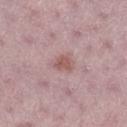{
  "biopsy_status": "not biopsied; imaged during a skin examination",
  "site": "leg",
  "patient": {
    "sex": "female",
    "age_approx": 40
  },
  "image": {
    "source": "total-body photography crop",
    "field_of_view_mm": 15
  },
  "automated_metrics": {
    "area_mm2_approx": 4.0,
    "eccentricity": 0.7,
    "shape_asymmetry": 0.2,
    "border_irregularity_0_10": 2.0,
    "color_variation_0_10": 1.5,
    "peripheral_color_asymmetry": 0.5,
    "nevus_likeness_0_100": 15
  },
  "lighting": "white-light",
  "lesion_size": {
    "long_diameter_mm_approx": 2.5
  }
}